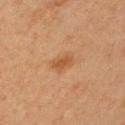This lesion was catalogued during total-body skin photography and was not selected for biopsy. This is a cross-polarized tile. A male subject approximately 60 years of age. A lesion tile, about 15 mm wide, cut from a 3D total-body photograph. Longest diameter approximately 3 mm. On the right upper arm.